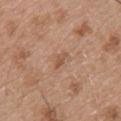| field | value |
|---|---|
| follow-up | catalogued during a skin exam; not biopsied |
| lesion size | ~2.5 mm (longest diameter) |
| automated lesion analysis | a mean CIELAB color near L≈53 a*≈21 b*≈32, a lesion–skin lightness drop of about 8, and a normalized border contrast of about 6; a classifier nevus-likeness of about 0/100 and a detector confidence of about 100 out of 100 that the crop contains a lesion |
| location | the back |
| subject | male, about 50 years old |
| imaging modality | 15 mm crop, total-body photography |